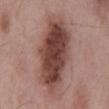Imaged with white-light lighting. A male patient aged around 55. The lesion is on the mid back. The lesion's longest dimension is about 10.5 mm. A 15 mm close-up tile from a total-body photography series done for melanoma screening. Automated tile analysis of the lesion measured a footprint of about 37 mm² and an eccentricity of roughly 0.9. The software also gave about 16 CIELAB-L* units darker than the surrounding skin and a lesion-to-skin contrast of about 11.5 (normalized; higher = more distinct).